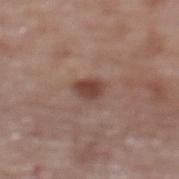biopsy_status: not biopsied; imaged during a skin examination
image:
  source: total-body photography crop
  field_of_view_mm: 15
lesion_size:
  long_diameter_mm_approx: 3.0
site: upper back
automated_metrics:
  area_mm2_approx: 4.5
  shape_asymmetry: 0.3
  cielab_L: 43
  cielab_a: 21
  cielab_b: 24
  vs_skin_contrast_norm: 9.0
  lesion_detection_confidence_0_100: 100
patient:
  sex: female
  age_approx: 65
lighting: white-light